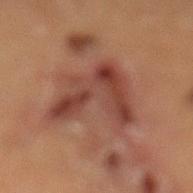Clinical impression:
This lesion was catalogued during total-body skin photography and was not selected for biopsy.
Image and clinical context:
The lesion is located on the left lower leg. This is a cross-polarized tile. The subject is a male approximately 60 years of age. The lesion-visualizer software estimated a footprint of about 24 mm² and a symmetry-axis asymmetry near 0.55. The analysis additionally found a lesion color around L≈35 a*≈20 b*≈23 in CIELAB, a lesion–skin lightness drop of about 8, and a normalized border contrast of about 8. And it measured border irregularity of about 7 on a 0–10 scale, a color-variation rating of about 5.5/10, and radial color variation of about 2. A 15 mm close-up extracted from a 3D total-body photography capture. Approximately 6.5 mm at its widest.